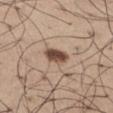follow-up: no biopsy performed (imaged during a skin exam) | illumination: white-light | subject: male, in their mid- to late 60s | acquisition: total-body-photography crop, ~15 mm field of view | location: the left thigh.